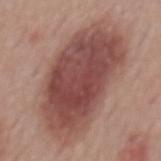From the back. A male subject in their mid-50s. A region of skin cropped from a whole-body photographic capture, roughly 15 mm wide. Approximately 11.5 mm at its widest.